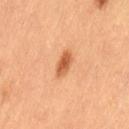Impression:
The lesion was photographed on a routine skin check and not biopsied; there is no pathology result.
Acquisition and patient details:
A close-up tile cropped from a whole-body skin photograph, about 15 mm across. Captured under cross-polarized illumination. On the left thigh. A female patient aged around 60. Longest diameter approximately 3.5 mm.